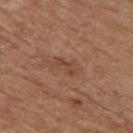| key | value |
|---|---|
| workup | catalogued during a skin exam; not biopsied |
| image source | ~15 mm tile from a whole-body skin photo |
| location | the upper back |
| lesion diameter | ≈3 mm |
| patient | male, aged 73 to 77 |